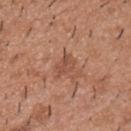Image and clinical context: Located on the back. Approximately 2.5 mm at its widest. The subject is a male aged 38–42. A roughly 15 mm field-of-view crop from a total-body skin photograph. Automated tile analysis of the lesion measured a lesion area of about 3.5 mm², a shape eccentricity near 0.7, and two-axis asymmetry of about 0.3. The software also gave an automated nevus-likeness rating near 0 out of 100 and lesion-presence confidence of about 100/100. Imaged with white-light lighting.